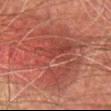No biopsy was performed on this lesion — it was imaged during a full skin examination and was not determined to be concerning. Cropped from a whole-body photographic skin survey; the tile spans about 15 mm. A male patient in their mid- to late 70s. Located on the chest.